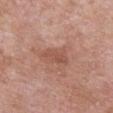workup — imaged on a skin check; not biopsied
subject — female, in their 70s
tile lighting — white-light illumination
diameter — about 3.5 mm
acquisition — 15 mm crop, total-body photography
anatomic site — the chest